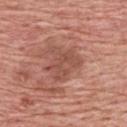{"site": "upper back", "image": {"source": "total-body photography crop", "field_of_view_mm": 15}, "patient": {"sex": "male", "age_approx": 60}, "automated_metrics": {"shape_asymmetry": 0.5, "nevus_likeness_0_100": 0, "lesion_detection_confidence_0_100": 100}, "lighting": "white-light"}